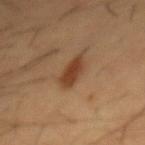No biopsy was performed on this lesion — it was imaged during a full skin examination and was not determined to be concerning.